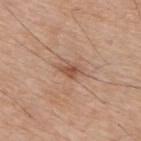Background: From the upper back. A male patient in their mid-70s. The recorded lesion diameter is about 2.5 mm. Cropped from a total-body skin-imaging series; the visible field is about 15 mm.